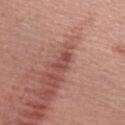Background: Located on the right upper arm. A 15 mm close-up extracted from a 3D total-body photography capture. The lesion's longest dimension is about 3 mm. Automated tile analysis of the lesion measured a lesion area of about 4 mm² and a symmetry-axis asymmetry near 0.3. The software also gave a lesion color around L≈48 a*≈26 b*≈25 in CIELAB and about 10 CIELAB-L* units darker than the surrounding skin. It also reported a border-irregularity rating of about 3.5/10 and peripheral color asymmetry of about 0.5. It also reported lesion-presence confidence of about 90/100. A female subject aged around 50.